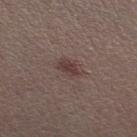- workup · catalogued during a skin exam; not biopsied
- site · the left thigh
- image · total-body-photography crop, ~15 mm field of view
- lesion size · about 2.5 mm
- lighting · white-light
- subject · female, about 30 years old